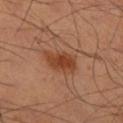Clinical impression:
Imaged during a routine full-body skin examination; the lesion was not biopsied and no histopathology is available.
Background:
A 15 mm crop from a total-body photograph taken for skin-cancer surveillance. A male subject, in their mid- to late 50s. Located on the right lower leg. Measured at roughly 4 mm in maximum diameter.